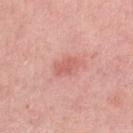notes = catalogued during a skin exam; not biopsied | imaging modality = total-body-photography crop, ~15 mm field of view | patient = female, aged approximately 40 | anatomic site = the left lower leg.